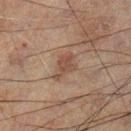  biopsy_status: not biopsied; imaged during a skin examination
  image:
    source: total-body photography crop
    field_of_view_mm: 15
  lighting: cross-polarized
  patient:
    sex: male
    age_approx: 60
  automated_metrics:
    area_mm2_approx: 5.5
    eccentricity: 0.8
    shape_asymmetry: 0.4
    cielab_L: 35
    cielab_a: 14
    cielab_b: 21
    vs_skin_darker_L: 7.0
    border_irregularity_0_10: 4.0
    color_variation_0_10: 2.0
    peripheral_color_asymmetry: 0.5
  lesion_size:
    long_diameter_mm_approx: 3.5
  site: left lower leg